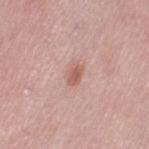  biopsy_status: not biopsied; imaged during a skin examination
  image:
    source: total-body photography crop
    field_of_view_mm: 15
  lesion_size:
    long_diameter_mm_approx: 2.5
  patient:
    sex: female
    age_approx: 50
  lighting: white-light
  site: left thigh